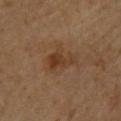– location: the upper back
– lesion diameter: about 4 mm
– illumination: cross-polarized
– image: ~15 mm crop, total-body skin-cancer survey
– subject: male, in their mid-80s or older